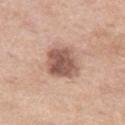location — the left thigh | automated lesion analysis — a shape eccentricity near 0.4 and two-axis asymmetry of about 0.15 | image — ~15 mm crop, total-body skin-cancer survey | subject — female, in their mid-50s | diameter — about 4 mm.